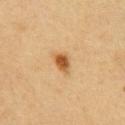- follow-up · catalogued during a skin exam; not biopsied
- imaging modality · total-body-photography crop, ~15 mm field of view
- lighting · cross-polarized illumination
- anatomic site · the arm
- patient · female, aged 28–32
- automated metrics · a lesion area of about 5 mm² and a shape-asymmetry score of about 0.3 (0 = symmetric); border irregularity of about 2.5 on a 0–10 scale, a color-variation rating of about 5/10, and peripheral color asymmetry of about 1.5; a classifier nevus-likeness of about 100/100 and a lesion-detection confidence of about 100/100
- lesion diameter · ~3 mm (longest diameter)